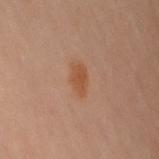follow-up: imaged on a skin check; not biopsied | subject: female, roughly 60 years of age | automated metrics: roughly 7 lightness units darker than nearby skin; a classifier nevus-likeness of about 95/100 | illumination: cross-polarized illumination | anatomic site: the arm | imaging modality: 15 mm crop, total-body photography.